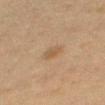Case summary:
- acquisition: 15 mm crop, total-body photography
- body site: the right thigh
- subject: female, roughly 55 years of age